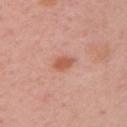Recorded during total-body skin imaging; not selected for excision or biopsy.
A close-up tile cropped from a whole-body skin photograph, about 15 mm across.
A female subject aged approximately 35.
Located on the arm.